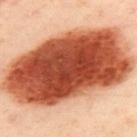An algorithmic analysis of the crop reported a mean CIELAB color near L≈55 a*≈32 b*≈38, about 26 CIELAB-L* units darker than the surrounding skin, and a normalized lesion–skin contrast near 15.5. It also reported a within-lesion color-variation index near 9.5/10. And it measured a classifier nevus-likeness of about 100/100 and a lesion-detection confidence of about 100/100. The lesion is on the upper back. A female subject aged approximately 40. Cropped from a whole-body photographic skin survey; the tile spans about 15 mm. Longest diameter approximately 15.5 mm. Captured under cross-polarized illumination.